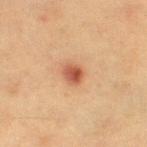Recorded during total-body skin imaging; not selected for excision or biopsy.